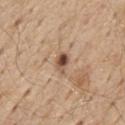{
  "biopsy_status": "not biopsied; imaged during a skin examination",
  "patient": {
    "sex": "male",
    "age_approx": 70
  },
  "site": "chest",
  "automated_metrics": {
    "area_mm2_approx": 4.0,
    "eccentricity": 0.75,
    "shape_asymmetry": 0.2,
    "cielab_L": 51,
    "cielab_a": 19,
    "cielab_b": 29,
    "vs_skin_contrast_norm": 9.5,
    "border_irregularity_0_10": 2.0
  },
  "image": {
    "source": "total-body photography crop",
    "field_of_view_mm": 15
  },
  "lighting": "white-light"
}